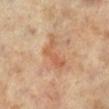The lesion was tiled from a total-body skin photograph and was not biopsied.